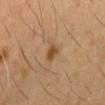{"biopsy_status": "not biopsied; imaged during a skin examination", "patient": {"sex": "male", "age_approx": 60}, "site": "left thigh", "image": {"source": "total-body photography crop", "field_of_view_mm": 15}, "lighting": "cross-polarized", "automated_metrics": {"cielab_L": 44, "cielab_a": 20, "cielab_b": 34, "vs_skin_darker_L": 10.0, "vs_skin_contrast_norm": 8.5}}